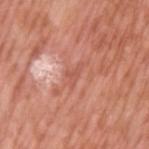workup = catalogued during a skin exam; not biopsied